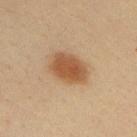<lesion>
  <biopsy_status>not biopsied; imaged during a skin examination</biopsy_status>
  <site>upper back</site>
  <image>
    <source>total-body photography crop</source>
    <field_of_view_mm>15</field_of_view_mm>
  </image>
  <automated_metrics>
    <cielab_L>48</cielab_L>
    <cielab_a>18</cielab_a>
    <cielab_b>32</cielab_b>
    <vs_skin_darker_L>11.0</vs_skin_darker_L>
    <vs_skin_contrast_norm>8.5</vs_skin_contrast_norm>
    <border_irregularity_0_10>1.5</border_irregularity_0_10>
    <color_variation_0_10>3.5</color_variation_0_10>
    <peripheral_color_asymmetry>1.0</peripheral_color_asymmetry>
  </automated_metrics>
  <patient>
    <sex>male</sex>
    <age_approx>35</age_approx>
  </patient>
</lesion>